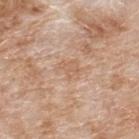| key | value |
|---|---|
| notes | total-body-photography surveillance lesion; no biopsy |
| subject | male, aged around 80 |
| body site | the upper back |
| image source | ~15 mm tile from a whole-body skin photo |